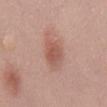| field | value |
|---|---|
| site | the mid back |
| image | ~15 mm tile from a whole-body skin photo |
| subject | male, approximately 35 years of age |